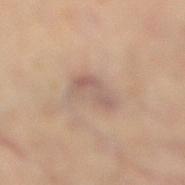Q: Was a biopsy performed?
A: catalogued during a skin exam; not biopsied
Q: What are the patient's age and sex?
A: female, approximately 60 years of age
Q: Where on the body is the lesion?
A: the right lower leg
Q: What is the imaging modality?
A: total-body-photography crop, ~15 mm field of view
Q: Illumination type?
A: cross-polarized illumination